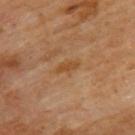Assessment:
Imaged during a routine full-body skin examination; the lesion was not biopsied and no histopathology is available.
Context:
Imaged with cross-polarized lighting. A 15 mm close-up extracted from a 3D total-body photography capture. A male subject aged 58–62. Located on the back.